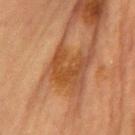<record>
<biopsy_status>not biopsied; imaged during a skin examination</biopsy_status>
<patient>
  <sex>female</sex>
  <age_approx>60</age_approx>
</patient>
<image>
  <source>total-body photography crop</source>
  <field_of_view_mm>15</field_of_view_mm>
</image>
<lighting>cross-polarized</lighting>
<automated_metrics>
  <area_mm2_approx>8.0</area_mm2_approx>
  <cielab_L>42</cielab_L>
  <cielab_a>23</cielab_a>
  <cielab_b>36</cielab_b>
  <vs_skin_darker_L>7.0</vs_skin_darker_L>
  <vs_skin_contrast_norm>6.5</vs_skin_contrast_norm>
  <border_irregularity_0_10>10.0</border_irregularity_0_10>
  <color_variation_0_10>1.5</color_variation_0_10>
  <peripheral_color_asymmetry>0.5</peripheral_color_asymmetry>
  <nevus_likeness_0_100>0</nevus_likeness_0_100>
  <lesion_detection_confidence_0_100>90</lesion_detection_confidence_0_100>
</automated_metrics>
<site>chest</site>
<lesion_size>
  <long_diameter_mm_approx>4.0</long_diameter_mm_approx>
</lesion_size>
</record>